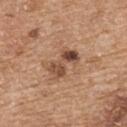{"biopsy_status": "not biopsied; imaged during a skin examination", "patient": {"sex": "female", "age_approx": 70}, "automated_metrics": {"area_mm2_approx": 7.5, "eccentricity": 0.9, "shape_asymmetry": 0.3, "border_irregularity_0_10": 4.5, "color_variation_0_10": 9.5, "peripheral_color_asymmetry": 3.0, "nevus_likeness_0_100": 15, "lesion_detection_confidence_0_100": 100}, "lesion_size": {"long_diameter_mm_approx": 4.0}, "image": {"source": "total-body photography crop", "field_of_view_mm": 15}, "site": "upper back", "lighting": "white-light"}